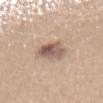Impression:
The lesion was tiled from a total-body skin photograph and was not biopsied.
Context:
A lesion tile, about 15 mm wide, cut from a 3D total-body photograph. From the abdomen. About 4.5 mm across. The total-body-photography lesion software estimated a lesion area of about 9 mm², a shape eccentricity near 0.8, and two-axis asymmetry of about 0.25. The software also gave a lesion color around L≈57 a*≈16 b*≈25 in CIELAB and a normalized border contrast of about 8. The analysis additionally found a classifier nevus-likeness of about 65/100 and a detector confidence of about 100 out of 100 that the crop contains a lesion. The patient is a female in their mid-40s. Captured under white-light illumination.